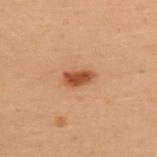Image and clinical context:
A female patient aged 48 to 52. From the upper back. Automated tile analysis of the lesion measured a footprint of about 5 mm² and an eccentricity of roughly 0.85. It also reported a mean CIELAB color near L≈44 a*≈23 b*≈34, about 12 CIELAB-L* units darker than the surrounding skin, and a lesion-to-skin contrast of about 9.5 (normalized; higher = more distinct). And it measured an automated nevus-likeness rating near 100 out of 100 and a lesion-detection confidence of about 100/100. A close-up tile cropped from a whole-body skin photograph, about 15 mm across.